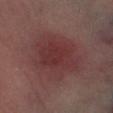Q: Is there a histopathology result?
A: no biopsy performed (imaged during a skin exam)
Q: How large is the lesion?
A: about 7 mm
Q: Patient demographics?
A: female, roughly 50 years of age
Q: What is the anatomic site?
A: the left lower leg
Q: What kind of image is this?
A: ~15 mm tile from a whole-body skin photo
Q: What did automated image analysis measure?
A: a lesion area of about 31 mm², a shape eccentricity near 0.5, and a shape-asymmetry score of about 0.2 (0 = symmetric); a lesion color around L≈33 a*≈22 b*≈18 in CIELAB, about 6 CIELAB-L* units darker than the surrounding skin, and a normalized border contrast of about 6.5; a nevus-likeness score of about 5/100 and a detector confidence of about 100 out of 100 that the crop contains a lesion